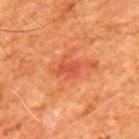  biopsy_status: not biopsied; imaged during a skin examination
  lighting: cross-polarized
  site: back
  automated_metrics:
    area_mm2_approx: 3.5
    eccentricity: 0.8
    cielab_L: 42
    cielab_a: 31
    cielab_b: 33
    vs_skin_darker_L: 7.0
    vs_skin_contrast_norm: 5.5
    border_irregularity_0_10: 3.5
    peripheral_color_asymmetry: 0.5
  image:
    source: total-body photography crop
    field_of_view_mm: 15
  patient:
    sex: male
    age_approx: 80